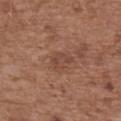The lesion was tiled from a total-body skin photograph and was not biopsied.
Measured at roughly 2.5 mm in maximum diameter.
A 15 mm close-up extracted from a 3D total-body photography capture.
The subject is a female approximately 75 years of age.
On the upper back.
An algorithmic analysis of the crop reported a lesion area of about 3.5 mm², an eccentricity of roughly 0.75, and two-axis asymmetry of about 0.45. The analysis additionally found an average lesion color of about L≈44 a*≈21 b*≈26 (CIELAB) and a normalized lesion–skin contrast near 5.5. And it measured peripheral color asymmetry of about 0.5. The software also gave a classifier nevus-likeness of about 0/100 and a lesion-detection confidence of about 100/100.
The tile uses white-light illumination.